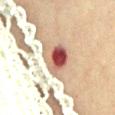Clinical impression:
Imaged during a routine full-body skin examination; the lesion was not biopsied and no histopathology is available.
Image and clinical context:
A roughly 15 mm field-of-view crop from a total-body skin photograph. Imaged with cross-polarized lighting. An algorithmic analysis of the crop reported an area of roughly 14 mm², an outline eccentricity of about 0.7 (0 = round, 1 = elongated), and a shape-asymmetry score of about 0.25 (0 = symmetric). It also reported a border-irregularity index near 2.5/10 and internal color variation of about 9 on a 0–10 scale. The analysis additionally found an automated nevus-likeness rating near 0 out of 100 and a detector confidence of about 100 out of 100 that the crop contains a lesion. From the abdomen. Measured at roughly 5.5 mm in maximum diameter. A female subject aged approximately 70.